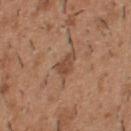| key | value |
|---|---|
| automated metrics | an average lesion color of about L≈47 a*≈20 b*≈31 (CIELAB), roughly 9 lightness units darker than nearby skin, and a normalized lesion–skin contrast near 6.5; a border-irregularity rating of about 4/10 and radial color variation of about 0.5; a nevus-likeness score of about 10/100 and a detector confidence of about 100 out of 100 that the crop contains a lesion |
| anatomic site | the upper back |
| acquisition | total-body-photography crop, ~15 mm field of view |
| lighting | white-light illumination |
| lesion diameter | about 3 mm |
| patient | male, aged 38 to 42 |